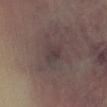biopsy status — catalogued during a skin exam; not biopsied
subject — in their mid-50s
site — the right lower leg
TBP lesion metrics — a footprint of about 4.5 mm² and a shape-asymmetry score of about 0.15 (0 = symmetric); an average lesion color of about L≈35 a*≈13 b*≈12 (CIELAB) and about 5 CIELAB-L* units darker than the surrounding skin; a within-lesion color-variation index near 2.5/10 and peripheral color asymmetry of about 1
lighting — cross-polarized
image source — ~15 mm tile from a whole-body skin photo